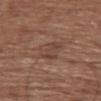{"biopsy_status": "not biopsied; imaged during a skin examination", "image": {"source": "total-body photography crop", "field_of_view_mm": 15}, "site": "head or neck", "patient": {"sex": "female", "age_approx": 75}, "automated_metrics": {"border_irregularity_0_10": 3.0, "peripheral_color_asymmetry": 1.0}, "lighting": "white-light"}